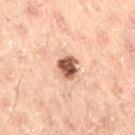biopsy status — catalogued during a skin exam; not biopsied | image — ~15 mm tile from a whole-body skin photo | patient — male, roughly 45 years of age | site — the right thigh.